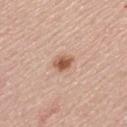notes = imaged on a skin check; not biopsied | lesion size = ~2.5 mm (longest diameter) | site = the mid back | tile lighting = white-light illumination | acquisition = total-body-photography crop, ~15 mm field of view | subject = male, roughly 60 years of age | automated metrics = an area of roughly 3.5 mm² and two-axis asymmetry of about 0.15; a color-variation rating of about 3/10 and a peripheral color-asymmetry measure near 1.